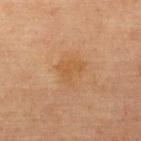Clinical impression: Part of a total-body skin-imaging series; this lesion was reviewed on a skin check and was not flagged for biopsy. Acquisition and patient details: The total-body-photography lesion software estimated a footprint of about 6 mm². And it measured a classifier nevus-likeness of about 0/100 and a lesion-detection confidence of about 100/100. About 3 mm across. A female patient, aged 63 to 67. A roughly 15 mm field-of-view crop from a total-body skin photograph. The tile uses cross-polarized illumination. From the upper back.